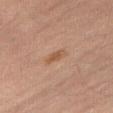Recorded during total-body skin imaging; not selected for excision or biopsy.
An algorithmic analysis of the crop reported a lesion color around L≈49 a*≈19 b*≈30 in CIELAB, about 8 CIELAB-L* units darker than the surrounding skin, and a normalized lesion–skin contrast near 6.5.
The recorded lesion diameter is about 3 mm.
A lesion tile, about 15 mm wide, cut from a 3D total-body photograph.
From the right thigh.
Imaged with cross-polarized lighting.
A male subject aged approximately 60.